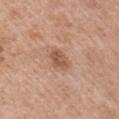<tbp_lesion>
  <biopsy_status>not biopsied; imaged during a skin examination</biopsy_status>
  <patient>
    <sex>male</sex>
    <age_approx>55</age_approx>
  </patient>
  <lighting>white-light</lighting>
  <lesion_size>
    <long_diameter_mm_approx>3.0</long_diameter_mm_approx>
  </lesion_size>
  <image>
    <source>total-body photography crop</source>
    <field_of_view_mm>15</field_of_view_mm>
  </image>
  <site>right upper arm</site>
</tbp_lesion>